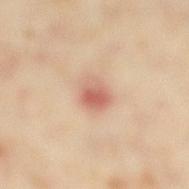Notes:
- imaging modality — ~15 mm crop, total-body skin-cancer survey
- lesion diameter — ~3 mm (longest diameter)
- location — the leg
- lighting — cross-polarized
- patient — female, aged around 35
- automated metrics — an area of roughly 7 mm², an outline eccentricity of about 0.55 (0 = round, 1 = elongated), and a symmetry-axis asymmetry near 0.15; a mean CIELAB color near L≈60 a*≈22 b*≈29, about 11 CIELAB-L* units darker than the surrounding skin, and a normalized lesion–skin contrast near 7; border irregularity of about 1.5 on a 0–10 scale, a color-variation rating of about 7.5/10, and radial color variation of about 2.5